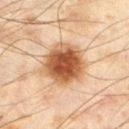Part of a total-body skin-imaging series; this lesion was reviewed on a skin check and was not flagged for biopsy. The subject is a male aged 43–47. A 15 mm close-up tile from a total-body photography series done for melanoma screening. This is a cross-polarized tile. The lesion is located on the left thigh.